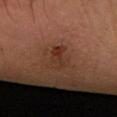workup: total-body-photography surveillance lesion; no biopsy
lesion size: about 4 mm
patient: male, aged 63 to 67
image: 15 mm crop, total-body photography
anatomic site: the left forearm
lighting: cross-polarized illumination
image-analysis metrics: an area of roughly 6.5 mm², an eccentricity of roughly 0.85, and a symmetry-axis asymmetry near 0.35; an average lesion color of about L≈33 a*≈21 b*≈28 (CIELAB) and a lesion-to-skin contrast of about 6.5 (normalized; higher = more distinct); a border-irregularity rating of about 4/10, internal color variation of about 8.5 on a 0–10 scale, and a peripheral color-asymmetry measure near 3.5; a lesion-detection confidence of about 100/100